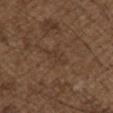lesion size = ≈2.5 mm | patient = male, in their 50s | imaging modality = total-body-photography crop, ~15 mm field of view | site = the chest.